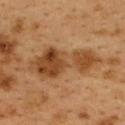Imaged during a routine full-body skin examination; the lesion was not biopsied and no histopathology is available. The subject is a female aged around 40. The recorded lesion diameter is about 9 mm. From the upper back. A close-up tile cropped from a whole-body skin photograph, about 15 mm across. Captured under cross-polarized illumination.